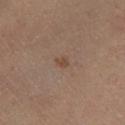No biopsy was performed on this lesion — it was imaged during a full skin examination and was not determined to be concerning. The lesion is on the right lower leg. Captured under cross-polarized illumination. Automated tile analysis of the lesion measured an area of roughly 2 mm², a shape eccentricity near 0.75, and a symmetry-axis asymmetry near 0.35. A female subject aged around 60. A 15 mm crop from a total-body photograph taken for skin-cancer surveillance. Longest diameter approximately 2 mm.